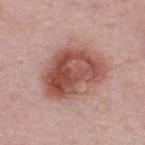This lesion was catalogued during total-body skin photography and was not selected for biopsy. Cropped from a whole-body photographic skin survey; the tile spans about 15 mm. The lesion is located on the upper back. A male patient roughly 50 years of age. Measured at roughly 7 mm in maximum diameter.